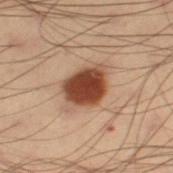{
  "patient": {
    "sex": "male",
    "age_approx": 55
  },
  "site": "leg",
  "lighting": "cross-polarized",
  "lesion_size": {
    "long_diameter_mm_approx": 4.5
  },
  "image": {
    "source": "total-body photography crop",
    "field_of_view_mm": 15
  }
}